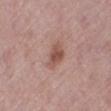Findings:
• workup — imaged on a skin check; not biopsied
• TBP lesion metrics — a mean CIELAB color near L≈52 a*≈22 b*≈25, roughly 10 lightness units darker than nearby skin, and a normalized lesion–skin contrast near 7.5
• lesion diameter — ~3.5 mm (longest diameter)
• image — ~15 mm crop, total-body skin-cancer survey
• subject — female, about 65 years old
• location — the left lower leg